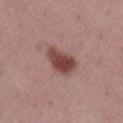{"biopsy_status": "not biopsied; imaged during a skin examination", "lesion_size": {"long_diameter_mm_approx": 4.0}, "automated_metrics": {"area_mm2_approx": 8.5, "shape_asymmetry": 0.25, "nevus_likeness_0_100": 100}, "site": "right lower leg", "patient": {"sex": "female", "age_approx": 55}, "image": {"source": "total-body photography crop", "field_of_view_mm": 15}, "lighting": "white-light"}